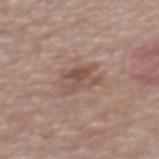Assessment:
The lesion was tiled from a total-body skin photograph and was not biopsied.
Clinical summary:
Measured at roughly 4 mm in maximum diameter. The subject is a male roughly 80 years of age. This is a white-light tile. This image is a 15 mm lesion crop taken from a total-body photograph. On the upper back.